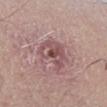Notes:
- notes · catalogued during a skin exam; not biopsied
- tile lighting · white-light illumination
- patient · male, aged around 55
- size · about 4 mm
- image-analysis metrics · a lesion area of about 10 mm² and a symmetry-axis asymmetry near 0.35; a lesion color around L≈51 a*≈22 b*≈18 in CIELAB, about 10 CIELAB-L* units darker than the surrounding skin, and a normalized lesion–skin contrast near 7; a border-irregularity index near 4.5/10, a color-variation rating of about 7.5/10, and a peripheral color-asymmetry measure near 2.5
- imaging modality · 15 mm crop, total-body photography
- location · the left lower leg